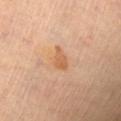The lesion was photographed on a routine skin check and not biopsied; there is no pathology result.
A male patient, about 60 years old.
Captured under cross-polarized illumination.
Automated image analysis of the tile measured roughly 8 lightness units darker than nearby skin and a lesion-to-skin contrast of about 6.5 (normalized; higher = more distinct). The software also gave a nevus-likeness score of about 30/100 and a detector confidence of about 100 out of 100 that the crop contains a lesion.
Approximately 3 mm at its widest.
From the chest.
Cropped from a total-body skin-imaging series; the visible field is about 15 mm.